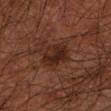| feature | finding |
|---|---|
| follow-up | total-body-photography surveillance lesion; no biopsy |
| anatomic site | the left forearm |
| patient | male, about 50 years old |
| lighting | cross-polarized |
| image | ~15 mm crop, total-body skin-cancer survey |
| diameter | ~3.5 mm (longest diameter) |